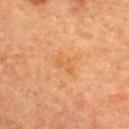Background:
A lesion tile, about 15 mm wide, cut from a 3D total-body photograph. The patient is a female in their mid- to late 50s. The recorded lesion diameter is about 2.5 mm. Captured under cross-polarized illumination. From the back.